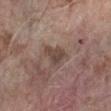<tbp_lesion>
<biopsy_status>not biopsied; imaged during a skin examination</biopsy_status>
<site>left forearm</site>
<automated_metrics>
  <area_mm2_approx>6.5</area_mm2_approx>
  <eccentricity>0.75</eccentricity>
  <shape_asymmetry>0.45</shape_asymmetry>
  <cielab_L>44</cielab_L>
  <cielab_a>15</cielab_a>
  <cielab_b>22</cielab_b>
  <vs_skin_contrast_norm>7.5</vs_skin_contrast_norm>
  <border_irregularity_0_10>4.5</border_irregularity_0_10>
  <color_variation_0_10>2.5</color_variation_0_10>
  <peripheral_color_asymmetry>1.0</peripheral_color_asymmetry>
</automated_metrics>
<lesion_size>
  <long_diameter_mm_approx>3.5</long_diameter_mm_approx>
</lesion_size>
<lighting>white-light</lighting>
<patient>
  <sex>male</sex>
  <age_approx>60</age_approx>
</patient>
<image>
  <source>total-body photography crop</source>
  <field_of_view_mm>15</field_of_view_mm>
</image>
</tbp_lesion>